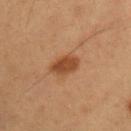Q: Was a biopsy performed?
A: no biopsy performed (imaged during a skin exam)
Q: Patient demographics?
A: male, aged around 55
Q: Where on the body is the lesion?
A: the chest
Q: How was this image acquired?
A: total-body-photography crop, ~15 mm field of view